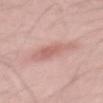Background: The total-body-photography lesion software estimated a lesion-to-skin contrast of about 5.5 (normalized; higher = more distinct). It also reported a nevus-likeness score of about 10/100 and a lesion-detection confidence of about 100/100. This is a white-light tile. The lesion is on the mid back. A male subject, roughly 30 years of age. A roughly 15 mm field-of-view crop from a total-body skin photograph.